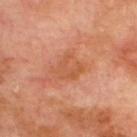Assessment: The lesion was photographed on a routine skin check and not biopsied; there is no pathology result. Acquisition and patient details: A male subject aged 68 to 72. Located on the upper back. A 15 mm crop from a total-body photograph taken for skin-cancer surveillance. Captured under cross-polarized illumination. Longest diameter approximately 3 mm.